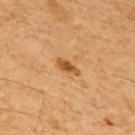Q: Was this lesion biopsied?
A: catalogued during a skin exam; not biopsied
Q: Where on the body is the lesion?
A: the mid back
Q: Automated lesion metrics?
A: an area of roughly 3 mm², an eccentricity of roughly 0.9, and two-axis asymmetry of about 0.25; an average lesion color of about L≈43 a*≈21 b*≈37 (CIELAB), a lesion–skin lightness drop of about 10, and a normalized lesion–skin contrast near 8.5
Q: How was the tile lit?
A: cross-polarized
Q: Patient demographics?
A: male, aged 58 to 62
Q: What is the lesion's diameter?
A: ~3 mm (longest diameter)
Q: How was this image acquired?
A: ~15 mm crop, total-body skin-cancer survey